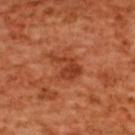workup: imaged on a skin check; not biopsied | imaging modality: total-body-photography crop, ~15 mm field of view | lesion size: ≈4 mm | TBP lesion metrics: a lesion area of about 6 mm², an outline eccentricity of about 0.8 (0 = round, 1 = elongated), and two-axis asymmetry of about 0.55; a lesion color around L≈42 a*≈32 b*≈38 in CIELAB | site: the upper back | tile lighting: cross-polarized | patient: female, in their mid-50s.